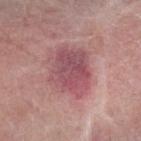Clinical impression:
The lesion was photographed on a routine skin check and not biopsied; there is no pathology result.
Image and clinical context:
The lesion is on the arm. Approximately 6 mm at its widest. This image is a 15 mm lesion crop taken from a total-body photograph. The tile uses white-light illumination. A female subject, approximately 65 years of age.